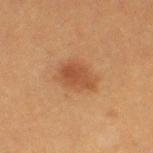Imaged during a routine full-body skin examination; the lesion was not biopsied and no histopathology is available.
A close-up tile cropped from a whole-body skin photograph, about 15 mm across.
Automated image analysis of the tile measured an outline eccentricity of about 0.8 (0 = round, 1 = elongated) and a shape-asymmetry score of about 0.25 (0 = symmetric). The analysis additionally found a border-irregularity rating of about 2.5/10, a color-variation rating of about 2.5/10, and radial color variation of about 1.
From the left thigh.
A female subject, roughly 40 years of age.
This is a cross-polarized tile.